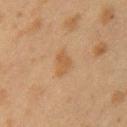<case>
  <biopsy_status>not biopsied; imaged during a skin examination</biopsy_status>
  <site>left upper arm</site>
  <lighting>cross-polarized</lighting>
  <automated_metrics>
    <area_mm2_approx>4.0</area_mm2_approx>
    <eccentricity>0.75</eccentricity>
    <cielab_L>46</cielab_L>
    <cielab_a>16</cielab_a>
    <cielab_b>32</cielab_b>
    <vs_skin_darker_L>6.0</vs_skin_darker_L>
    <vs_skin_contrast_norm>6.0</vs_skin_contrast_norm>
    <color_variation_0_10>1.0</color_variation_0_10>
    <peripheral_color_asymmetry>0.5</peripheral_color_asymmetry>
    <nevus_likeness_0_100>25</nevus_likeness_0_100>
    <lesion_detection_confidence_0_100>100</lesion_detection_confidence_0_100>
  </automated_metrics>
  <lesion_size>
    <long_diameter_mm_approx>3.0</long_diameter_mm_approx>
  </lesion_size>
  <image>
    <source>total-body photography crop</source>
    <field_of_view_mm>15</field_of_view_mm>
  </image>
  <patient>
    <sex>female</sex>
    <age_approx>40</age_approx>
  </patient>
</case>